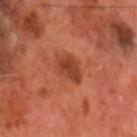Q: Was a biopsy performed?
A: total-body-photography surveillance lesion; no biopsy
Q: What is the lesion's diameter?
A: ≈3.5 mm
Q: What are the patient's age and sex?
A: male, aged 68 to 72
Q: Where on the body is the lesion?
A: the head or neck
Q: Illumination type?
A: cross-polarized illumination
Q: What is the imaging modality?
A: total-body-photography crop, ~15 mm field of view